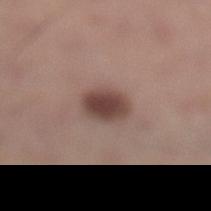follow-up: no biopsy performed (imaged during a skin exam) | patient: male, aged 53–57 | size: about 3.5 mm | image: ~15 mm crop, total-body skin-cancer survey | location: the left lower leg | automated metrics: a border-irregularity rating of about 1.5/10 | tile lighting: white-light.